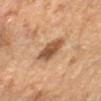notes: catalogued during a skin exam; not biopsied.